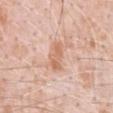Longest diameter approximately 3.5 mm. The subject is a male approximately 50 years of age. On the front of the torso. A 15 mm crop from a total-body photograph taken for skin-cancer surveillance. Captured under white-light illumination.